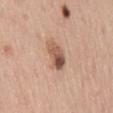Captured during whole-body skin photography for melanoma surveillance; the lesion was not biopsied. A 15 mm close-up tile from a total-body photography series done for melanoma screening. Located on the mid back. Longest diameter approximately 3.5 mm. A male patient, about 45 years old. Automated tile analysis of the lesion measured a shape eccentricity near 0.8 and a symmetry-axis asymmetry near 0.2. The software also gave a lesion color around L≈55 a*≈20 b*≈29 in CIELAB and a lesion-to-skin contrast of about 9 (normalized; higher = more distinct). It also reported a border-irregularity index near 2/10, a within-lesion color-variation index near 9.5/10, and a peripheral color-asymmetry measure near 4.5. The analysis additionally found a nevus-likeness score of about 75/100 and a detector confidence of about 100 out of 100 that the crop contains a lesion.